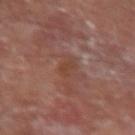Imaged with cross-polarized lighting.
A male patient, aged around 60.
Located on the right forearm.
A region of skin cropped from a whole-body photographic capture, roughly 15 mm wide.
The lesion-visualizer software estimated an average lesion color of about L≈40 a*≈20 b*≈27 (CIELAB), roughly 5 lightness units darker than nearby skin, and a normalized border contrast of about 6.5.
Approximately 3 mm at its widest.